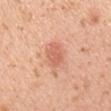| key | value |
|---|---|
| follow-up | imaged on a skin check; not biopsied |